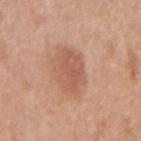This lesion was catalogued during total-body skin photography and was not selected for biopsy.
A close-up tile cropped from a whole-body skin photograph, about 15 mm across.
On the right upper arm.
The subject is a female roughly 50 years of age.